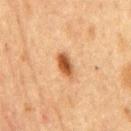Assessment:
Recorded during total-body skin imaging; not selected for excision or biopsy.
Clinical summary:
Imaged with cross-polarized lighting. The subject is a male aged approximately 75. A roughly 15 mm field-of-view crop from a total-body skin photograph. Approximately 3.5 mm at its widest. The lesion is on the chest.